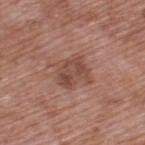{"biopsy_status": "not biopsied; imaged during a skin examination", "site": "upper back", "image": {"source": "total-body photography crop", "field_of_view_mm": 15}, "automated_metrics": {"area_mm2_approx": 11.0, "eccentricity": 0.5, "nevus_likeness_0_100": 10, "lesion_detection_confidence_0_100": 100}, "lesion_size": {"long_diameter_mm_approx": 4.0}, "patient": {"sex": "male", "age_approx": 70}}